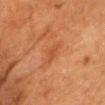Recorded during total-body skin imaging; not selected for excision or biopsy. A male subject aged around 70. On the chest. Automated tile analysis of the lesion measured a footprint of about 3 mm² and an outline eccentricity of about 0.8 (0 = round, 1 = elongated). It also reported a border-irregularity index near 3.5/10 and a color-variation rating of about 0.5/10. The software also gave a nevus-likeness score of about 0/100. Longest diameter approximately 2.5 mm. Imaged with cross-polarized lighting. Cropped from a total-body skin-imaging series; the visible field is about 15 mm.